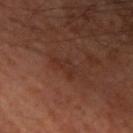notes = catalogued during a skin exam; not biopsied | diameter = ≈3.5 mm | image-analysis metrics = an automated nevus-likeness rating near 0 out of 100 and a detector confidence of about 100 out of 100 that the crop contains a lesion | image source = 15 mm crop, total-body photography | patient = male, in their mid- to late 60s | site = the left upper arm.